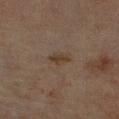Impression: Part of a total-body skin-imaging series; this lesion was reviewed on a skin check and was not flagged for biopsy. Background: Measured at roughly 3 mm in maximum diameter. The lesion is on the right upper arm. Automated tile analysis of the lesion measured an area of roughly 3 mm², an outline eccentricity of about 0.85 (0 = round, 1 = elongated), and a shape-asymmetry score of about 0.3 (0 = symmetric). The software also gave internal color variation of about 1.5 on a 0–10 scale. The analysis additionally found an automated nevus-likeness rating near 30 out of 100 and a lesion-detection confidence of about 100/100. This is a cross-polarized tile. A 15 mm close-up extracted from a 3D total-body photography capture. A male patient aged 68 to 72.